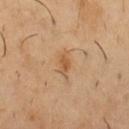Imaged during a routine full-body skin examination; the lesion was not biopsied and no histopathology is available. A lesion tile, about 15 mm wide, cut from a 3D total-body photograph. Imaged with cross-polarized lighting. Longest diameter approximately 2.5 mm. The subject is a male in their 50s. The lesion is located on the chest.